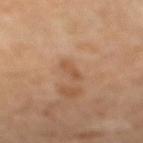{"biopsy_status": "not biopsied; imaged during a skin examination", "automated_metrics": {"area_mm2_approx": 3.0, "shape_asymmetry": 0.15, "peripheral_color_asymmetry": 0.0}, "site": "right lower leg", "image": {"source": "total-body photography crop", "field_of_view_mm": 15}, "patient": {"sex": "female", "age_approx": 70}, "lighting": "cross-polarized", "lesion_size": {"long_diameter_mm_approx": 2.5}}